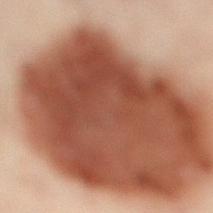Captured during whole-body skin photography for melanoma surveillance; the lesion was not biopsied. On the left leg. A male patient aged around 50. A roughly 15 mm field-of-view crop from a total-body skin photograph.